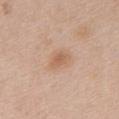A female patient in their 60s.
A 15 mm crop from a total-body photograph taken for skin-cancer surveillance.
Located on the back.